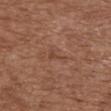| key | value |
|---|---|
| body site | the chest |
| subject | female, aged around 75 |
| lesion size | about 2.5 mm |
| automated metrics | an area of roughly 2.5 mm² and a shape eccentricity near 0.85 |
| lighting | white-light |
| acquisition | ~15 mm crop, total-body skin-cancer survey |